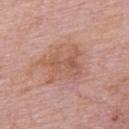biopsy status = imaged on a skin check; not biopsied
patient = male, in their mid-70s
illumination = white-light illumination
image = ~15 mm crop, total-body skin-cancer survey
body site = the upper back
lesion diameter = about 5.5 mm
automated metrics = a normalized border contrast of about 5.5; an automated nevus-likeness rating near 5 out of 100 and a lesion-detection confidence of about 100/100